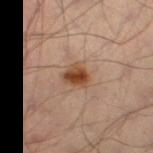This lesion was catalogued during total-body skin photography and was not selected for biopsy.
Automated image analysis of the tile measured an area of roughly 6 mm², an eccentricity of roughly 0.55, and a shape-asymmetry score of about 0.2 (0 = symmetric). The analysis additionally found a within-lesion color-variation index near 6/10 and radial color variation of about 2. The analysis additionally found a nevus-likeness score of about 95/100 and lesion-presence confidence of about 100/100.
A male subject, approximately 50 years of age.
Approximately 3 mm at its widest.
From the leg.
A 15 mm crop from a total-body photograph taken for skin-cancer surveillance.
The tile uses cross-polarized illumination.